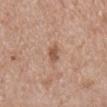Imaged during a routine full-body skin examination; the lesion was not biopsied and no histopathology is available. On the mid back. This image is a 15 mm lesion crop taken from a total-body photograph. The patient is a male aged approximately 60. An algorithmic analysis of the crop reported a shape eccentricity near 0.9 and a symmetry-axis asymmetry near 0.2. The analysis additionally found a within-lesion color-variation index near 1/10 and a peripheral color-asymmetry measure near 0. Captured under white-light illumination.